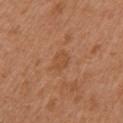Part of a total-body skin-imaging series; this lesion was reviewed on a skin check and was not flagged for biopsy. A lesion tile, about 15 mm wide, cut from a 3D total-body photograph. A female subject aged approximately 40. The tile uses white-light illumination. Automated tile analysis of the lesion measured a shape eccentricity near 0.65 and a symmetry-axis asymmetry near 0.2. The analysis additionally found a border-irregularity index near 2/10 and a color-variation rating of about 1/10. And it measured a classifier nevus-likeness of about 0/100 and lesion-presence confidence of about 100/100. From the chest.